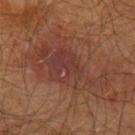Recorded during total-body skin imaging; not selected for excision or biopsy.
Automated tile analysis of the lesion measured an area of roughly 36 mm², an outline eccentricity of about 0.85 (0 = round, 1 = elongated), and a symmetry-axis asymmetry near 0.45. It also reported a nevus-likeness score of about 20/100 and lesion-presence confidence of about 100/100.
Approximately 10.5 mm at its widest.
A region of skin cropped from a whole-body photographic capture, roughly 15 mm wide.
Located on the left upper arm.
The tile uses cross-polarized illumination.
A male patient, aged 63–67.